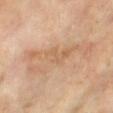| key | value |
|---|---|
| follow-up | imaged on a skin check; not biopsied |
| subject | female, aged 73 to 77 |
| imaging modality | total-body-photography crop, ~15 mm field of view |
| lesion diameter | about 9 mm |
| anatomic site | the right thigh |
| tile lighting | cross-polarized |